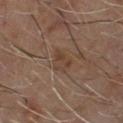| key | value |
|---|---|
| biopsy status | no biopsy performed (imaged during a skin exam) |
| location | the chest |
| illumination | cross-polarized illumination |
| automated metrics | a footprint of about 5 mm², a shape eccentricity near 0.65, and a shape-asymmetry score of about 0.4 (0 = symmetric); an average lesion color of about L≈37 a*≈15 b*≈24 (CIELAB), a lesion–skin lightness drop of about 5, and a lesion-to-skin contrast of about 5.5 (normalized; higher = more distinct); a nevus-likeness score of about 0/100 and a lesion-detection confidence of about 100/100 |
| lesion size | ≈3 mm |
| patient | male, about 60 years old |
| imaging modality | ~15 mm tile from a whole-body skin photo |